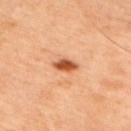The lesion was photographed on a routine skin check and not biopsied; there is no pathology result.
Imaged with cross-polarized lighting.
The lesion is on the right upper arm.
A male patient aged 43–47.
A close-up tile cropped from a whole-body skin photograph, about 15 mm across.
The lesion's longest dimension is about 3 mm.